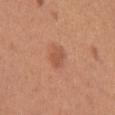biopsy_status: not biopsied; imaged during a skin examination
lesion_size:
  long_diameter_mm_approx: 2.5
automated_metrics:
  area_mm2_approx: 4.0
  eccentricity: 0.75
  shape_asymmetry: 0.15
  cielab_L: 54
  cielab_a: 26
  cielab_b: 34
  vs_skin_darker_L: 8.0
  vs_skin_contrast_norm: 6.0
  border_irregularity_0_10: 1.5
  color_variation_0_10: 1.5
  peripheral_color_asymmetry: 0.5
patient:
  sex: female
  age_approx: 25
site: right upper arm
lighting: white-light
image:
  source: total-body photography crop
  field_of_view_mm: 15